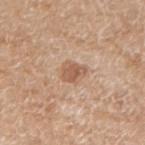Impression:
Recorded during total-body skin imaging; not selected for excision or biopsy.
Image and clinical context:
Captured under white-light illumination. A close-up tile cropped from a whole-body skin photograph, about 15 mm across. The lesion is on the left forearm. Longest diameter approximately 3 mm. A female patient in their mid- to late 70s. An algorithmic analysis of the crop reported a footprint of about 4.5 mm², an eccentricity of roughly 0.7, and a symmetry-axis asymmetry near 0.25.